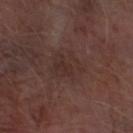Recorded during total-body skin imaging; not selected for excision or biopsy. Imaged with white-light lighting. About 4 mm across. A male subject, in their 70s. From the right forearm. A 15 mm crop from a total-body photograph taken for skin-cancer surveillance.